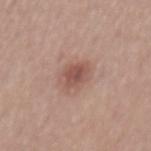workup: no biopsy performed (imaged during a skin exam) | lighting: white-light illumination | imaging modality: ~15 mm tile from a whole-body skin photo | patient: male, roughly 55 years of age | diameter: about 3 mm | location: the mid back | TBP lesion metrics: a footprint of about 5.5 mm²; a border-irregularity rating of about 2/10 and a peripheral color-asymmetry measure near 1.